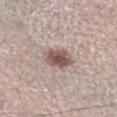Clinical impression: Recorded during total-body skin imaging; not selected for excision or biopsy. Context: A male patient about 60 years old. Automated image analysis of the tile measured an average lesion color of about L≈53 a*≈16 b*≈22 (CIELAB), about 14 CIELAB-L* units darker than the surrounding skin, and a lesion-to-skin contrast of about 10 (normalized; higher = more distinct). And it measured border irregularity of about 2 on a 0–10 scale, internal color variation of about 3 on a 0–10 scale, and radial color variation of about 1. The software also gave a classifier nevus-likeness of about 95/100 and lesion-presence confidence of about 100/100. Located on the right lower leg. This is a white-light tile. A region of skin cropped from a whole-body photographic capture, roughly 15 mm wide.